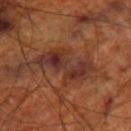Assessment:
Part of a total-body skin-imaging series; this lesion was reviewed on a skin check and was not flagged for biopsy.
Acquisition and patient details:
An algorithmic analysis of the crop reported a normalized border contrast of about 9.5. It also reported border irregularity of about 7 on a 0–10 scale, a color-variation rating of about 6.5/10, and radial color variation of about 2. The analysis additionally found an automated nevus-likeness rating near 0 out of 100 and lesion-presence confidence of about 65/100. Captured under cross-polarized illumination. A 15 mm close-up tile from a total-body photography series done for melanoma screening. The lesion's longest dimension is about 6.5 mm. The lesion is on the right thigh. A male subject roughly 70 years of age.